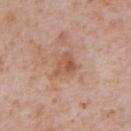Assessment:
The lesion was photographed on a routine skin check and not biopsied; there is no pathology result.
Acquisition and patient details:
A lesion tile, about 15 mm wide, cut from a 3D total-body photograph. The lesion is on the chest. This is a white-light tile. A male patient aged around 65.